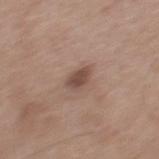  biopsy_status: not biopsied; imaged during a skin examination
  automated_metrics:
    border_irregularity_0_10: 2.0
    color_variation_0_10: 2.0
    nevus_likeness_0_100: 80
    lesion_detection_confidence_0_100: 100
  lesion_size:
    long_diameter_mm_approx: 2.5
  patient:
    sex: male
    age_approx: 55
  lighting: white-light
  site: mid back
  image:
    source: total-body photography crop
    field_of_view_mm: 15